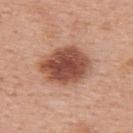Clinical summary:
The lesion's longest dimension is about 6 mm. A 15 mm close-up extracted from a 3D total-body photography capture. The lesion is on the upper back. A female patient, aged approximately 50. The tile uses white-light illumination.